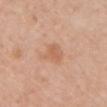{"biopsy_status": "not biopsied; imaged during a skin examination", "lesion_size": {"long_diameter_mm_approx": 3.0}, "patient": {"sex": "female", "age_approx": 65}, "lighting": "white-light", "image": {"source": "total-body photography crop", "field_of_view_mm": 15}, "automated_metrics": {"area_mm2_approx": 5.0, "eccentricity": 0.6, "shape_asymmetry": 0.35, "nevus_likeness_0_100": 0, "lesion_detection_confidence_0_100": 100}, "site": "chest"}